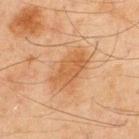Clinical impression: This lesion was catalogued during total-body skin photography and was not selected for biopsy.